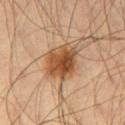biopsy status: imaged on a skin check; not biopsied